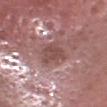The lesion was photographed on a routine skin check and not biopsied; there is no pathology result.